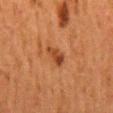{"patient": {"sex": "female", "age_approx": 50}, "image": {"source": "total-body photography crop", "field_of_view_mm": 15}, "automated_metrics": {"area_mm2_approx": 4.0, "eccentricity": 0.85, "shape_asymmetry": 0.25, "border_irregularity_0_10": 2.5, "color_variation_0_10": 3.5, "peripheral_color_asymmetry": 1.0}, "site": "back", "lesion_size": {"long_diameter_mm_approx": 3.0}, "lighting": "cross-polarized"}